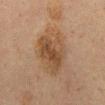No biopsy was performed on this lesion — it was imaged during a full skin examination and was not determined to be concerning. Imaged with cross-polarized lighting. On the front of the torso. A close-up tile cropped from a whole-body skin photograph, about 15 mm across. The recorded lesion diameter is about 7.5 mm. A male patient in their 60s. Automated tile analysis of the lesion measured an area of roughly 22 mm², a shape eccentricity near 0.85, and two-axis asymmetry of about 0.2. And it measured a border-irregularity rating of about 2.5/10, a within-lesion color-variation index near 5/10, and a peripheral color-asymmetry measure near 1.5.